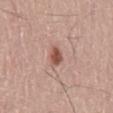Impression:
No biopsy was performed on this lesion — it was imaged during a full skin examination and was not determined to be concerning.
Context:
On the mid back. A lesion tile, about 15 mm wide, cut from a 3D total-body photograph. The recorded lesion diameter is about 2.5 mm. The subject is a male aged approximately 60.